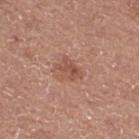This lesion was catalogued during total-body skin photography and was not selected for biopsy. The subject is a female aged 48 to 52. On the right thigh. A region of skin cropped from a whole-body photographic capture, roughly 15 mm wide. The lesion-visualizer software estimated a lesion area of about 4 mm² and an outline eccentricity of about 0.8 (0 = round, 1 = elongated). The recorded lesion diameter is about 3 mm. Imaged with white-light lighting.